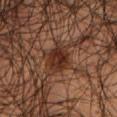notes = catalogued during a skin exam; not biopsied
patient = male, aged approximately 60
site = the front of the torso
image source = 15 mm crop, total-body photography